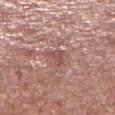The lesion was photographed on a routine skin check and not biopsied; there is no pathology result.